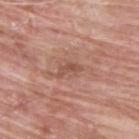<lesion>
<image>
  <source>total-body photography crop</source>
  <field_of_view_mm>15</field_of_view_mm>
</image>
<patient>
  <sex>male</sex>
  <age_approx>65</age_approx>
</patient>
<site>upper back</site>
<automated_metrics>
  <cielab_L>51</cielab_L>
  <cielab_a>23</cielab_a>
  <cielab_b>28</cielab_b>
  <vs_skin_darker_L>8.0</vs_skin_darker_L>
  <vs_skin_contrast_norm>6.0</vs_skin_contrast_norm>
  <border_irregularity_0_10>5.0</border_irregularity_0_10>
  <color_variation_0_10>0.0</color_variation_0_10>
  <peripheral_color_asymmetry>0.0</peripheral_color_asymmetry>
  <nevus_likeness_0_100>0</nevus_likeness_0_100>
  <lesion_detection_confidence_0_100>100</lesion_detection_confidence_0_100>
</automated_metrics>
<lighting>white-light</lighting>
<lesion_size>
  <long_diameter_mm_approx>3.0</long_diameter_mm_approx>
</lesion_size>
</lesion>